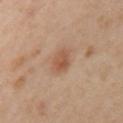Q: Was a biopsy performed?
A: catalogued during a skin exam; not biopsied
Q: What are the patient's age and sex?
A: female, roughly 40 years of age
Q: What is the anatomic site?
A: the left upper arm
Q: How was the tile lit?
A: cross-polarized illumination
Q: What is the lesion's diameter?
A: ~2.5 mm (longest diameter)
Q: How was this image acquired?
A: ~15 mm tile from a whole-body skin photo